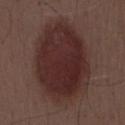Notes:
- workup: imaged on a skin check; not biopsied
- patient: male, aged around 55
- tile lighting: white-light
- location: the back
- imaging modality: ~15 mm tile from a whole-body skin photo
- TBP lesion metrics: an outline eccentricity of about 0.75 (0 = round, 1 = elongated) and a symmetry-axis asymmetry near 0.1; lesion-presence confidence of about 100/100